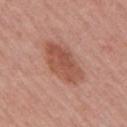follow-up = total-body-photography surveillance lesion; no biopsy
image source = 15 mm crop, total-body photography
illumination = white-light
patient = female, aged approximately 55
site = the right upper arm